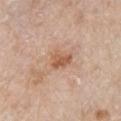* follow-up — total-body-photography surveillance lesion; no biopsy
* lighting — white-light
* image source — 15 mm crop, total-body photography
* patient — male, approximately 80 years of age
* location — the right upper arm